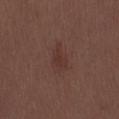Part of a total-body skin-imaging series; this lesion was reviewed on a skin check and was not flagged for biopsy. From the back. The patient is a male aged 28–32. Imaged with white-light lighting. A 15 mm crop from a total-body photograph taken for skin-cancer surveillance. The lesion's longest dimension is about 3.5 mm.